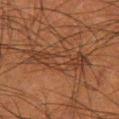This lesion was catalogued during total-body skin photography and was not selected for biopsy.
Cropped from a whole-body photographic skin survey; the tile spans about 15 mm.
An algorithmic analysis of the crop reported an eccentricity of roughly 0.95. And it measured a mean CIELAB color near L≈30 a*≈18 b*≈25 and a normalized lesion–skin contrast near 6.5. The software also gave a detector confidence of about 65 out of 100 that the crop contains a lesion.
The subject is a male aged approximately 50.
From the left thigh.
Captured under cross-polarized illumination.
Approximately 7 mm at its widest.